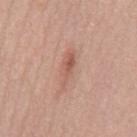{"biopsy_status": "not biopsied; imaged during a skin examination", "patient": {"sex": "male", "age_approx": 55}, "lesion_size": {"long_diameter_mm_approx": 4.5}, "image": {"source": "total-body photography crop", "field_of_view_mm": 15}, "automated_metrics": {"area_mm2_approx": 5.5, "eccentricity": 0.95, "shape_asymmetry": 0.3}, "site": "mid back", "lighting": "white-light"}